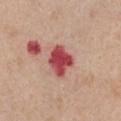The lesion was photographed on a routine skin check and not biopsied; there is no pathology result. Located on the chest. A lesion tile, about 15 mm wide, cut from a 3D total-body photograph. A female patient aged approximately 40.